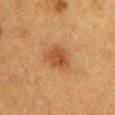Q: What kind of image is this?
A: ~15 mm crop, total-body skin-cancer survey
Q: What lighting was used for the tile?
A: cross-polarized
Q: Lesion size?
A: ~3.5 mm (longest diameter)
Q: Who is the patient?
A: female, aged 38–42
Q: Automated lesion metrics?
A: a mean CIELAB color near L≈41 a*≈22 b*≈33 and roughly 8 lightness units darker than nearby skin; a border-irregularity rating of about 3/10, internal color variation of about 3 on a 0–10 scale, and peripheral color asymmetry of about 1
Q: Lesion location?
A: the chest